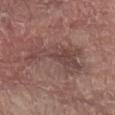Findings:
• biopsy status — total-body-photography surveillance lesion; no biopsy
• size — ~7.5 mm (longest diameter)
• image — 15 mm crop, total-body photography
• patient — male, approximately 80 years of age
• anatomic site — the abdomen
• automated metrics — an average lesion color of about L≈44 a*≈19 b*≈20 (CIELAB), a lesion–skin lightness drop of about 7, and a normalized lesion–skin contrast near 6